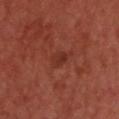lesion size = ≈2.5 mm
lighting = cross-polarized illumination
body site = the head or neck
patient = male, aged approximately 65
acquisition = ~15 mm crop, total-body skin-cancer survey